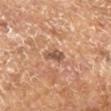Background: This image is a 15 mm lesion crop taken from a total-body photograph. Measured at roughly 2.5 mm in maximum diameter. The subject is a male in their mid-60s. This is a cross-polarized tile.